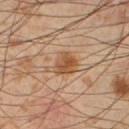workup: imaged on a skin check; not biopsied
diameter: ~3.5 mm (longest diameter)
image source: total-body-photography crop, ~15 mm field of view
TBP lesion metrics: an area of roughly 6.5 mm² and a shape-asymmetry score of about 0.4 (0 = symmetric); a classifier nevus-likeness of about 80/100
site: the left lower leg
illumination: cross-polarized illumination
subject: male, about 55 years old